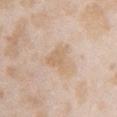<tbp_lesion>
  <biopsy_status>not biopsied; imaged during a skin examination</biopsy_status>
</tbp_lesion>